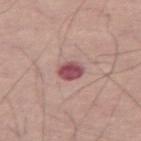  biopsy_status: not biopsied; imaged during a skin examination
  image:
    source: total-body photography crop
    field_of_view_mm: 15
  site: right thigh
  lesion_size:
    long_diameter_mm_approx: 2.5
  patient:
    sex: male
    age_approx: 65
  automated_metrics:
    area_mm2_approx: 5.5
    eccentricity: 0.35
    border_irregularity_0_10: 1.0
    color_variation_0_10: 4.0
    peripheral_color_asymmetry: 1.5
    lesion_detection_confidence_0_100: 100